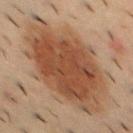{
  "biopsy_status": "not biopsied; imaged during a skin examination",
  "lesion_size": {
    "long_diameter_mm_approx": 10.0
  },
  "lighting": "cross-polarized",
  "patient": {
    "sex": "male",
    "age_approx": 55
  },
  "site": "upper back",
  "automated_metrics": {
    "area_mm2_approx": 55.0,
    "eccentricity": 0.65,
    "shape_asymmetry": 0.2,
    "cielab_L": 41,
    "cielab_a": 18,
    "cielab_b": 28,
    "vs_skin_darker_L": 11.0,
    "vs_skin_contrast_norm": 9.0,
    "lesion_detection_confidence_0_100": 100
  },
  "image": {
    "source": "total-body photography crop",
    "field_of_view_mm": 15
  }
}